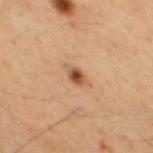Recorded during total-body skin imaging; not selected for excision or biopsy. From the mid back. A roughly 15 mm field-of-view crop from a total-body skin photograph. The total-body-photography lesion software estimated an average lesion color of about L≈49 a*≈21 b*≈33 (CIELAB) and a normalized border contrast of about 9.5. This is a cross-polarized tile. Approximately 2 mm at its widest. A male patient, aged approximately 50.